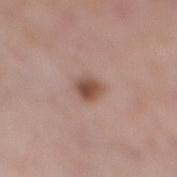{"biopsy_status": "not biopsied; imaged during a skin examination", "patient": {"sex": "female", "age_approx": 65}, "lesion_size": {"long_diameter_mm_approx": 2.5}, "lighting": "white-light", "image": {"source": "total-body photography crop", "field_of_view_mm": 15}, "site": "right lower leg", "automated_metrics": {"area_mm2_approx": 5.0, "eccentricity": 0.65, "shape_asymmetry": 0.25, "cielab_L": 50, "cielab_a": 20, "cielab_b": 26, "vs_skin_contrast_norm": 9.0, "peripheral_color_asymmetry": 1.5, "nevus_likeness_0_100": 95, "lesion_detection_confidence_0_100": 100}}